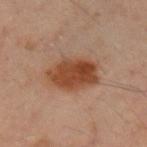Image and clinical context:
An algorithmic analysis of the crop reported a lesion area of about 17 mm² and two-axis asymmetry of about 0.15. And it measured a mean CIELAB color near L≈40 a*≈20 b*≈29, a lesion–skin lightness drop of about 11, and a lesion-to-skin contrast of about 9.5 (normalized; higher = more distinct). And it measured a nevus-likeness score of about 100/100 and a lesion-detection confidence of about 100/100. A lesion tile, about 15 mm wide, cut from a 3D total-body photograph. The patient is a male aged around 50. On the left upper arm.